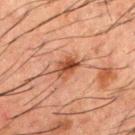This lesion was catalogued during total-body skin photography and was not selected for biopsy. A close-up tile cropped from a whole-body skin photograph, about 15 mm across. From the mid back. The subject is a male aged 48 to 52. The total-body-photography lesion software estimated a lesion area of about 5 mm², an eccentricity of roughly 0.75, and a shape-asymmetry score of about 0.25 (0 = symmetric). The tile uses cross-polarized illumination. The lesion's longest dimension is about 3 mm.